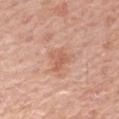patient = female, approximately 65 years of age
body site = the right forearm
automated lesion analysis = a lesion color around L≈60 a*≈25 b*≈31 in CIELAB, about 8 CIELAB-L* units darker than the surrounding skin, and a normalized lesion–skin contrast near 5.5; an automated nevus-likeness rating near 15 out of 100 and a lesion-detection confidence of about 100/100
lesion size = ~3 mm (longest diameter)
imaging modality = 15 mm crop, total-body photography